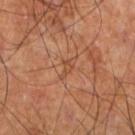The lesion was tiled from a total-body skin photograph and was not biopsied.
The lesion is on the left lower leg.
A 15 mm close-up tile from a total-body photography series done for melanoma screening.
A male patient, aged approximately 60.